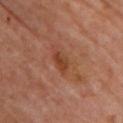A female subject aged 58–62. A 15 mm crop from a total-body photograph taken for skin-cancer surveillance. The lesion is on the chest.